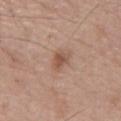biopsy status=catalogued during a skin exam; not biopsied
patient=male, aged 73–77
site=the front of the torso
tile lighting=white-light illumination
diameter=about 2.5 mm
image source=total-body-photography crop, ~15 mm field of view